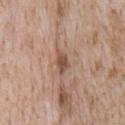biopsy status: no biopsy performed (imaged during a skin exam) | acquisition: total-body-photography crop, ~15 mm field of view | anatomic site: the front of the torso | tile lighting: white-light illumination | lesion size: about 3 mm | patient: male, aged 63 to 67 | automated metrics: a within-lesion color-variation index near 2.5/10 and a peripheral color-asymmetry measure near 0.5; an automated nevus-likeness rating near 35 out of 100.